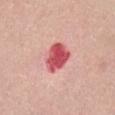The lesion was tiled from a total-body skin photograph and was not biopsied.
The subject is a female aged approximately 55.
Automated image analysis of the tile measured a shape eccentricity near 0.65 and a symmetry-axis asymmetry near 0.25. The analysis additionally found border irregularity of about 2 on a 0–10 scale, a within-lesion color-variation index near 4.5/10, and a peripheral color-asymmetry measure near 1.5.
A 15 mm close-up extracted from a 3D total-body photography capture.
The recorded lesion diameter is about 4 mm.
Located on the abdomen.
This is a white-light tile.